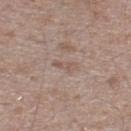Recorded during total-body skin imaging; not selected for excision or biopsy.
A roughly 15 mm field-of-view crop from a total-body skin photograph.
Approximately 2.5 mm at its widest.
Captured under white-light illumination.
The lesion is located on the leg.
The subject is a male in their mid- to late 40s.